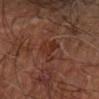Captured under cross-polarized illumination. The subject is a male roughly 70 years of age. Cropped from a whole-body photographic skin survey; the tile spans about 15 mm. On the arm.